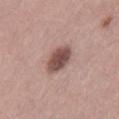No biopsy was performed on this lesion — it was imaged during a full skin examination and was not determined to be concerning.
A female subject approximately 40 years of age.
About 4 mm across.
The lesion is on the lower back.
A region of skin cropped from a whole-body photographic capture, roughly 15 mm wide.